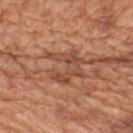<lesion>
  <biopsy_status>not biopsied; imaged during a skin examination</biopsy_status>
  <patient>
    <sex>male</sex>
    <age_approx>65</age_approx>
  </patient>
  <automated_metrics>
    <area_mm2_approx>10.0</area_mm2_approx>
    <eccentricity>0.45</eccentricity>
    <border_irregularity_0_10>9.0</border_irregularity_0_10>
    <color_variation_0_10>4.5</color_variation_0_10>
    <peripheral_color_asymmetry>1.5</peripheral_color_asymmetry>
    <nevus_likeness_0_100>0</nevus_likeness_0_100>
    <lesion_detection_confidence_0_100>65</lesion_detection_confidence_0_100>
  </automated_metrics>
  <site>mid back</site>
  <lesion_size>
    <long_diameter_mm_approx>4.5</long_diameter_mm_approx>
  </lesion_size>
  <image>
    <source>total-body photography crop</source>
    <field_of_view_mm>15</field_of_view_mm>
  </image>
</lesion>